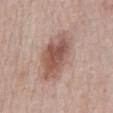biopsy status — imaged on a skin check; not biopsied
lighting — white-light
diameter — about 7 mm
subject — male, roughly 70 years of age
site — the abdomen
imaging modality — ~15 mm tile from a whole-body skin photo